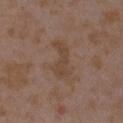biopsy status: total-body-photography surveillance lesion; no biopsy | illumination: white-light | size: about 5 mm | imaging modality: ~15 mm crop, total-body skin-cancer survey | subject: female, aged around 35 | site: the left upper arm.